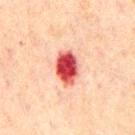- follow-up: catalogued during a skin exam; not biopsied
- subject: male, in their 60s
- acquisition: 15 mm crop, total-body photography
- site: the chest